The total-body-photography lesion software estimated a border-irregularity index near 1/10, a within-lesion color-variation index near 4/10, and a peripheral color-asymmetry measure near 1 · the patient is a male aged 48–52 · located on the abdomen · cropped from a whole-body photographic skin survey; the tile spans about 15 mm · the tile uses white-light illumination: 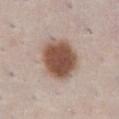Diagnosis: The lesion was biopsied, and histopathology showed a compound melanocytic nevus, classified as a benign lesion.